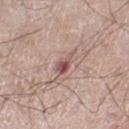– notes — no biopsy performed (imaged during a skin exam)
– image — ~15 mm crop, total-body skin-cancer survey
– lesion size — about 3 mm
– tile lighting — white-light
– anatomic site — the left thigh
– subject — male, aged around 70